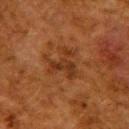Q: Who is the patient?
A: female, aged 48–52
Q: Lesion location?
A: the back
Q: How large is the lesion?
A: ≈4 mm
Q: Illumination type?
A: cross-polarized
Q: What kind of image is this?
A: total-body-photography crop, ~15 mm field of view